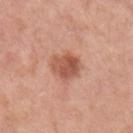Q: Was this lesion biopsied?
A: imaged on a skin check; not biopsied
Q: What is the imaging modality?
A: total-body-photography crop, ~15 mm field of view
Q: What did automated image analysis measure?
A: a lesion color around L≈55 a*≈26 b*≈31 in CIELAB, about 12 CIELAB-L* units darker than the surrounding skin, and a lesion-to-skin contrast of about 8 (normalized; higher = more distinct)
Q: What is the anatomic site?
A: the left upper arm
Q: What is the lesion's diameter?
A: about 4 mm
Q: How was the tile lit?
A: white-light illumination
Q: Who is the patient?
A: female, roughly 60 years of age